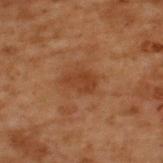Notes:
– lesion diameter — about 3.5 mm
– location — the upper back
– subject — male, roughly 70 years of age
– TBP lesion metrics — an outline eccentricity of about 0.8 (0 = round, 1 = elongated); a border-irregularity index near 3/10, internal color variation of about 2 on a 0–10 scale, and radial color variation of about 1; a nevus-likeness score of about 0/100 and a lesion-detection confidence of about 100/100
– illumination — cross-polarized illumination
– acquisition — ~15 mm tile from a whole-body skin photo